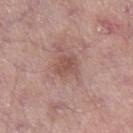No biopsy was performed on this lesion — it was imaged during a full skin examination and was not determined to be concerning. Located on the right thigh. A 15 mm close-up tile from a total-body photography series done for melanoma screening. This is a white-light tile. Measured at roughly 3 mm in maximum diameter. A male subject, roughly 80 years of age.